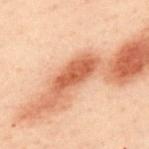workup — total-body-photography surveillance lesion; no biopsy
size — about 5.5 mm
TBP lesion metrics — a shape eccentricity near 0.95 and a symmetry-axis asymmetry near 0.3; a border-irregularity rating of about 3.5/10, a color-variation rating of about 2.5/10, and peripheral color asymmetry of about 1; an automated nevus-likeness rating near 45 out of 100 and a detector confidence of about 100 out of 100 that the crop contains a lesion
subject — male, approximately 50 years of age
site — the upper back
illumination — cross-polarized
acquisition — 15 mm crop, total-body photography